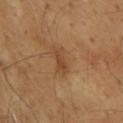Acquisition and patient details: A 15 mm close-up extracted from a 3D total-body photography capture. On the upper back. A male patient about 65 years old.